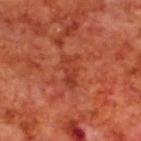| key | value |
|---|---|
| biopsy status | no biopsy performed (imaged during a skin exam) |
| patient | male, in their 70s |
| imaging modality | ~15 mm crop, total-body skin-cancer survey |
| body site | the upper back |